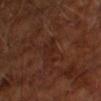Impression:
The lesion was tiled from a total-body skin photograph and was not biopsied.
Image and clinical context:
The lesion's longest dimension is about 3.5 mm. Cropped from a whole-body photographic skin survey; the tile spans about 15 mm. An algorithmic analysis of the crop reported a lesion color around L≈22 a*≈19 b*≈23 in CIELAB, about 4 CIELAB-L* units darker than the surrounding skin, and a normalized lesion–skin contrast near 5. A male patient, in their mid-60s. Imaged with cross-polarized lighting. On the left upper arm.